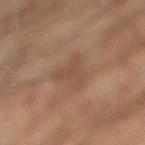- workup: imaged on a skin check; not biopsied
- image-analysis metrics: an average lesion color of about L≈35 a*≈15 b*≈21 (CIELAB) and roughly 5 lightness units darker than nearby skin; a border-irregularity rating of about 7/10, internal color variation of about 2 on a 0–10 scale, and a peripheral color-asymmetry measure near 0.5; a classifier nevus-likeness of about 0/100 and a lesion-detection confidence of about 100/100
- patient: male, roughly 60 years of age
- site: the left leg
- imaging modality: ~15 mm tile from a whole-body skin photo
- diameter: ~5 mm (longest diameter)
- illumination: cross-polarized illumination